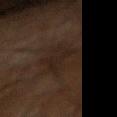An algorithmic analysis of the crop reported an area of roughly 10 mm², an eccentricity of roughly 0.8, and a shape-asymmetry score of about 0.35 (0 = symmetric). The software also gave border irregularity of about 5.5 on a 0–10 scale and a within-lesion color-variation index near 2/10. Imaged with cross-polarized lighting. From the left forearm. A lesion tile, about 15 mm wide, cut from a 3D total-body photograph. A male patient aged approximately 60. Measured at roughly 5.5 mm in maximum diameter.